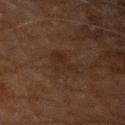Impression: Imaged during a routine full-body skin examination; the lesion was not biopsied and no histopathology is available. Context: A close-up tile cropped from a whole-body skin photograph, about 15 mm across. The total-body-photography lesion software estimated a border-irregularity rating of about 6/10, internal color variation of about 2 on a 0–10 scale, and radial color variation of about 0.5. The software also gave an automated nevus-likeness rating near 0 out of 100 and a lesion-detection confidence of about 100/100. The lesion is located on the left forearm. A male subject approximately 60 years of age. This is a cross-polarized tile.